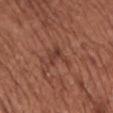Notes:
– site · the chest
– image · ~15 mm crop, total-body skin-cancer survey
– subject · female, approximately 75 years of age
– lesion size · ≈3 mm
– illumination · white-light